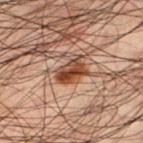The lesion was tiled from a total-body skin photograph and was not biopsied.
A roughly 15 mm field-of-view crop from a total-body skin photograph.
A male patient approximately 50 years of age.
The recorded lesion diameter is about 3.5 mm.
From the left lower leg.